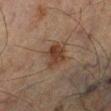This lesion was catalogued during total-body skin photography and was not selected for biopsy. A 15 mm crop from a total-body photograph taken for skin-cancer surveillance. On the right lower leg. A male subject aged 63–67. Imaged with cross-polarized lighting. Approximately 3 mm at its widest.